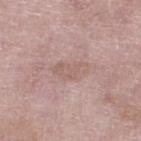Recorded during total-body skin imaging; not selected for excision or biopsy.
On the leg.
The tile uses white-light illumination.
A region of skin cropped from a whole-body photographic capture, roughly 15 mm wide.
The subject is a female in their 70s.
About 4.5 mm across.